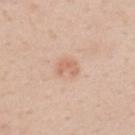The lesion's longest dimension is about 3 mm. A male patient aged 48–52. Cropped from a total-body skin-imaging series; the visible field is about 15 mm. The lesion is on the upper back. This is a white-light tile.